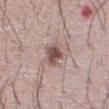Imaged during a routine full-body skin examination; the lesion was not biopsied and no histopathology is available. About 3 mm across. Automated tile analysis of the lesion measured a shape eccentricity near 0.65 and a symmetry-axis asymmetry near 0.25. The software also gave a within-lesion color-variation index near 4/10 and peripheral color asymmetry of about 1.5. It also reported a classifier nevus-likeness of about 80/100 and lesion-presence confidence of about 100/100. A male subject about 65 years old. The lesion is on the abdomen. Captured under white-light illumination. A close-up tile cropped from a whole-body skin photograph, about 15 mm across.